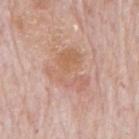follow-up: imaged on a skin check; not biopsied
image: ~15 mm crop, total-body skin-cancer survey
illumination: white-light illumination
site: the mid back
TBP lesion metrics: an area of roughly 14 mm² and an outline eccentricity of about 0.7 (0 = round, 1 = elongated); a border-irregularity index near 6.5/10 and internal color variation of about 5.5 on a 0–10 scale
subject: male, aged around 80
lesion diameter: ≈5 mm